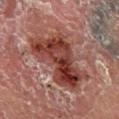The lesion was tiled from a total-body skin photograph and was not biopsied. A male subject, about 55 years old. On the right lower leg. This image is a 15 mm lesion crop taken from a total-body photograph.